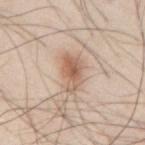Acquisition and patient details:
A male patient, in their mid- to late 40s. Automated tile analysis of the lesion measured a lesion area of about 8.5 mm² and an eccentricity of roughly 0.8. The software also gave a mean CIELAB color near L≈61 a*≈18 b*≈30, about 11 CIELAB-L* units darker than the surrounding skin, and a lesion-to-skin contrast of about 7.5 (normalized; higher = more distinct). The lesion is on the mid back. A 15 mm close-up extracted from a 3D total-body photography capture.